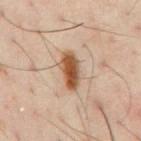Recorded during total-body skin imaging; not selected for excision or biopsy.